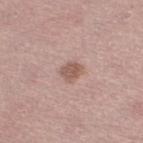Assessment:
The lesion was tiled from a total-body skin photograph and was not biopsied.
Acquisition and patient details:
Imaged with white-light lighting. Located on the left lower leg. The total-body-photography lesion software estimated a border-irregularity index near 1.5/10, a within-lesion color-variation index near 2/10, and radial color variation of about 1. The software also gave a detector confidence of about 100 out of 100 that the crop contains a lesion. Approximately 2.5 mm at its widest. A female patient, in their mid-40s. A 15 mm close-up extracted from a 3D total-body photography capture.